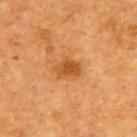<tbp_lesion>
<biopsy_status>not biopsied; imaged during a skin examination</biopsy_status>
<lighting>cross-polarized</lighting>
<site>upper back</site>
<patient>
  <sex>male</sex>
  <age_approx>75</age_approx>
</patient>
<automated_metrics>
  <area_mm2_approx>5.5</area_mm2_approx>
  <eccentricity>0.75</eccentricity>
  <shape_asymmetry>0.3</shape_asymmetry>
  <nevus_likeness_0_100>65</nevus_likeness_0_100>
</automated_metrics>
<lesion_size>
  <long_diameter_mm_approx>3.5</long_diameter_mm_approx>
</lesion_size>
<image>
  <source>total-body photography crop</source>
  <field_of_view_mm>15</field_of_view_mm>
</image>
</tbp_lesion>